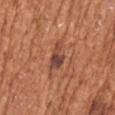notes — imaged on a skin check; not biopsied | image — ~15 mm tile from a whole-body skin photo | subject — female, aged 73 to 77 | body site — the arm.